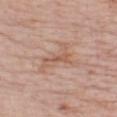Case summary:
- workup — imaged on a skin check; not biopsied
- location — the head or neck
- patient — male, aged around 75
- size — ≈5.5 mm
- acquisition — total-body-photography crop, ~15 mm field of view
- TBP lesion metrics — a footprint of about 9 mm², an outline eccentricity of about 0.9 (0 = round, 1 = elongated), and two-axis asymmetry of about 0.55; border irregularity of about 8.5 on a 0–10 scale, a within-lesion color-variation index near 3/10, and radial color variation of about 1; a nevus-likeness score of about 0/100 and a detector confidence of about 95 out of 100 that the crop contains a lesion
- tile lighting — white-light